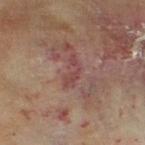{
  "biopsy_status": "not biopsied; imaged during a skin examination",
  "patient": {
    "sex": "female",
    "age_approx": 60
  },
  "image": {
    "source": "total-body photography crop",
    "field_of_view_mm": 15
  },
  "automated_metrics": {
    "vs_skin_darker_L": 6.0,
    "vs_skin_contrast_norm": 5.5,
    "peripheral_color_asymmetry": 0.0
  },
  "lighting": "cross-polarized",
  "site": "left thigh",
  "lesion_size": {
    "long_diameter_mm_approx": 4.0
  }
}